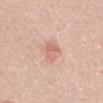follow-up: no biopsy performed (imaged during a skin exam) | lesion diameter: ≈3.5 mm | imaging modality: ~15 mm tile from a whole-body skin photo | subject: male, aged 48 to 52 | location: the front of the torso.